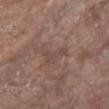<record>
  <biopsy_status>not biopsied; imaged during a skin examination</biopsy_status>
  <image>
    <source>total-body photography crop</source>
    <field_of_view_mm>15</field_of_view_mm>
  </image>
  <site>chest</site>
  <patient>
    <sex>female</sex>
    <age_approx>75</age_approx>
  </patient>
</record>